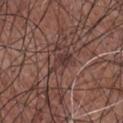The lesion was tiled from a total-body skin photograph and was not biopsied.
The lesion's longest dimension is about 3.5 mm.
A male subject roughly 75 years of age.
Automated tile analysis of the lesion measured a border-irregularity rating of about 5/10, internal color variation of about 2 on a 0–10 scale, and radial color variation of about 1. It also reported a nevus-likeness score of about 0/100 and a lesion-detection confidence of about 90/100.
Imaged with white-light lighting.
The lesion is on the chest.
A 15 mm crop from a total-body photograph taken for skin-cancer surveillance.